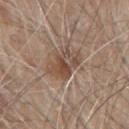biopsy status: no biopsy performed (imaged during a skin exam) | image-analysis metrics: a lesion–skin lightness drop of about 10 and a normalized lesion–skin contrast near 7.5; a border-irregularity rating of about 3/10 and internal color variation of about 7.5 on a 0–10 scale | image: ~15 mm tile from a whole-body skin photo | subject: male, about 80 years old | illumination: white-light | location: the mid back.